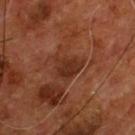{
  "image": {
    "source": "total-body photography crop",
    "field_of_view_mm": 15
  },
  "lighting": "cross-polarized",
  "site": "front of the torso",
  "patient": {
    "sex": "male",
    "age_approx": 55
  }
}